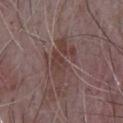Impression:
No biopsy was performed on this lesion — it was imaged during a full skin examination and was not determined to be concerning.
Clinical summary:
Approximately 7 mm at its widest. A male patient in their mid- to late 60s. This is a white-light tile. A 15 mm close-up extracted from a 3D total-body photography capture. On the chest.